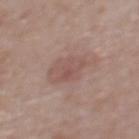Assessment:
Imaged during a routine full-body skin examination; the lesion was not biopsied and no histopathology is available.
Acquisition and patient details:
Captured under white-light illumination. The lesion is located on the back. A 15 mm close-up tile from a total-body photography series done for melanoma screening. The recorded lesion diameter is about 6 mm. A female subject, aged approximately 65.